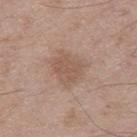Q: Was this lesion biopsied?
A: total-body-photography surveillance lesion; no biopsy
Q: What kind of image is this?
A: ~15 mm tile from a whole-body skin photo
Q: Lesion location?
A: the right thigh
Q: Patient demographics?
A: male, in their 50s